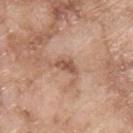No biopsy was performed on this lesion — it was imaged during a full skin examination and was not determined to be concerning. A lesion tile, about 15 mm wide, cut from a 3D total-body photograph. This is a white-light tile. Approximately 3 mm at its widest. A male patient, approximately 65 years of age. On the upper back.